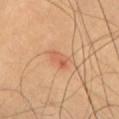{
  "biopsy_status": "not biopsied; imaged during a skin examination",
  "image": {
    "source": "total-body photography crop",
    "field_of_view_mm": 15
  },
  "automated_metrics": {
    "area_mm2_approx": 4.0,
    "eccentricity": 0.9,
    "shape_asymmetry": 0.25,
    "nevus_likeness_0_100": 5,
    "lesion_detection_confidence_0_100": 100
  },
  "lesion_size": {
    "long_diameter_mm_approx": 3.0
  },
  "lighting": "cross-polarized",
  "site": "chest"
}